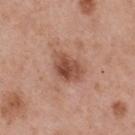| field | value |
|---|---|
| size | about 4.5 mm |
| location | the upper back |
| imaging modality | 15 mm crop, total-body photography |
| subject | male, aged approximately 55 |
| tile lighting | white-light |